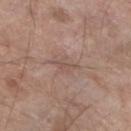Clinical impression: The lesion was photographed on a routine skin check and not biopsied; there is no pathology result. Acquisition and patient details: A male patient, aged 78–82. The lesion is located on the left lower leg. Cropped from a total-body skin-imaging series; the visible field is about 15 mm. Measured at roughly 3 mm in maximum diameter. The total-body-photography lesion software estimated a within-lesion color-variation index near 0/10 and radial color variation of about 0. And it measured a nevus-likeness score of about 0/100 and a detector confidence of about 90 out of 100 that the crop contains a lesion. Imaged with white-light lighting.